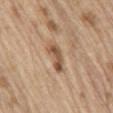Q: Was this lesion biopsied?
A: imaged on a skin check; not biopsied
Q: What are the patient's age and sex?
A: male, about 70 years old
Q: Where on the body is the lesion?
A: the mid back
Q: What kind of image is this?
A: ~15 mm tile from a whole-body skin photo
Q: What lighting was used for the tile?
A: white-light